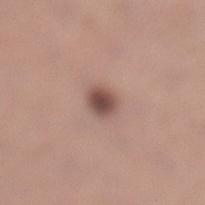Notes:
• workup: no biopsy performed (imaged during a skin exam)
• illumination: white-light
• patient: female, about 30 years old
• lesion size: ≈2.5 mm
• body site: the left lower leg
• image source: total-body-photography crop, ~15 mm field of view